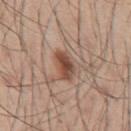biopsy status: catalogued during a skin exam; not biopsied.